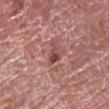This is a white-light tile. A female patient, aged approximately 60. A 15 mm close-up tile from a total-body photography series done for melanoma screening. The recorded lesion diameter is about 3 mm. The lesion is located on the right forearm.